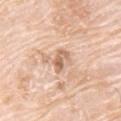notes: imaged on a skin check; not biopsied | subject: male, aged 73–77 | anatomic site: the upper back | image-analysis metrics: a lesion area of about 4 mm² and two-axis asymmetry of about 0.35; a mean CIELAB color near L≈65 a*≈20 b*≈32, roughly 12 lightness units darker than nearby skin, and a lesion-to-skin contrast of about 7.5 (normalized; higher = more distinct); a classifier nevus-likeness of about 0/100 and a detector confidence of about 100 out of 100 that the crop contains a lesion | illumination: white-light | acquisition: ~15 mm crop, total-body skin-cancer survey.